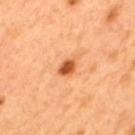Captured during whole-body skin photography for melanoma surveillance; the lesion was not biopsied. A male subject about 50 years old. About 3 mm across. Captured under cross-polarized illumination. Cropped from a whole-body photographic skin survey; the tile spans about 15 mm. Located on the mid back. The total-body-photography lesion software estimated border irregularity of about 2 on a 0–10 scale, a within-lesion color-variation index near 4.5/10, and radial color variation of about 1.5.